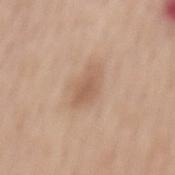Notes:
• notes · no biopsy performed (imaged during a skin exam)
• imaging modality · ~15 mm crop, total-body skin-cancer survey
• subject · female, aged around 65
• lighting · white-light illumination
• diameter · about 4 mm
• automated metrics · an automated nevus-likeness rating near 10 out of 100 and a detector confidence of about 100 out of 100 that the crop contains a lesion
• anatomic site · the back